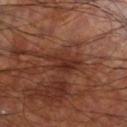<record>
<biopsy_status>not biopsied; imaged during a skin examination</biopsy_status>
<lesion_size>
  <long_diameter_mm_approx>4.5</long_diameter_mm_approx>
</lesion_size>
<lighting>cross-polarized</lighting>
<patient>
  <sex>male</sex>
  <age_approx>60</age_approx>
</patient>
<image>
  <source>total-body photography crop</source>
  <field_of_view_mm>15</field_of_view_mm>
</image>
<site>right lower leg</site>
<automated_metrics>
  <area_mm2_approx>6.0</area_mm2_approx>
  <eccentricity>0.85</eccentricity>
  <shape_asymmetry>0.35</shape_asymmetry>
  <border_irregularity_0_10>5.0</border_irregularity_0_10>
  <color_variation_0_10>3.0</color_variation_0_10>
  <peripheral_color_asymmetry>1.0</peripheral_color_asymmetry>
  <lesion_detection_confidence_0_100>85</lesion_detection_confidence_0_100>
</automated_metrics>
</record>